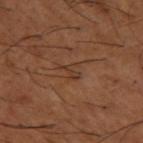biopsy status: total-body-photography surveillance lesion; no biopsy
anatomic site: the left thigh
image source: ~15 mm crop, total-body skin-cancer survey
subject: male, aged approximately 65
diameter: ≈2.5 mm
automated metrics: a lesion area of about 3.5 mm², a shape eccentricity near 0.7, and two-axis asymmetry of about 0.55; an average lesion color of about L≈36 a*≈19 b*≈29 (CIELAB), a lesion–skin lightness drop of about 6, and a normalized lesion–skin contrast near 5.5; a classifier nevus-likeness of about 0/100 and a lesion-detection confidence of about 95/100
lighting: cross-polarized